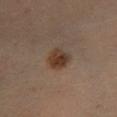biopsy status: catalogued during a skin exam; not biopsied | TBP lesion metrics: a footprint of about 7 mm² and an outline eccentricity of about 0.4 (0 = round, 1 = elongated); a border-irregularity rating of about 2/10 and a color-variation rating of about 4.5/10 | imaging modality: ~15 mm tile from a whole-body skin photo | anatomic site: the right lower leg | subject: male, in their 60s | lesion diameter: ≈3 mm | lighting: cross-polarized.